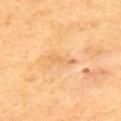workup: imaged on a skin check; not biopsied | illumination: cross-polarized | diameter: about 3.5 mm | anatomic site: the upper back | subject: male, roughly 55 years of age | image: total-body-photography crop, ~15 mm field of view.